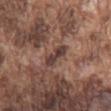biopsy status: total-body-photography surveillance lesion; no biopsy | patient: male, aged around 75 | tile lighting: white-light | diameter: ≈3.5 mm | site: the chest | acquisition: ~15 mm crop, total-body skin-cancer survey.